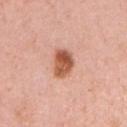Captured during whole-body skin photography for melanoma surveillance; the lesion was not biopsied.
From the right upper arm.
The recorded lesion diameter is about 3.5 mm.
Cropped from a total-body skin-imaging series; the visible field is about 15 mm.
A female patient in their mid- to late 30s.
Imaged with white-light lighting.
The total-body-photography lesion software estimated a classifier nevus-likeness of about 100/100 and a lesion-detection confidence of about 100/100.